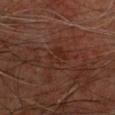<record>
<biopsy_status>not biopsied; imaged during a skin examination</biopsy_status>
<site>back</site>
<patient>
  <sex>male</sex>
  <age_approx>60</age_approx>
</patient>
<lighting>cross-polarized</lighting>
<automated_metrics>
  <area_mm2_approx>5.5</area_mm2_approx>
  <eccentricity>0.8</eccentricity>
  <border_irregularity_0_10>7.0</border_irregularity_0_10>
  <peripheral_color_asymmetry>0.5</peripheral_color_asymmetry>
</automated_metrics>
<image>
  <source>total-body photography crop</source>
  <field_of_view_mm>15</field_of_view_mm>
</image>
<lesion_size>
  <long_diameter_mm_approx>4.0</long_diameter_mm_approx>
</lesion_size>
</record>